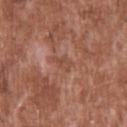biopsy status — no biopsy performed (imaged during a skin exam) | body site — the upper back | patient — male, aged approximately 45 | image source — 15 mm crop, total-body photography | lighting — white-light | image-analysis metrics — a footprint of about 3.5 mm², an eccentricity of roughly 0.8, and two-axis asymmetry of about 0.45; border irregularity of about 5 on a 0–10 scale, a within-lesion color-variation index near 1.5/10, and radial color variation of about 0.5; a classifier nevus-likeness of about 0/100 and a lesion-detection confidence of about 90/100 | size — about 3 mm.